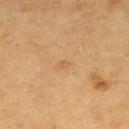Impression:
This lesion was catalogued during total-body skin photography and was not selected for biopsy.
Acquisition and patient details:
This is a cross-polarized tile. The patient is a female roughly 60 years of age. On the back. This image is a 15 mm lesion crop taken from a total-body photograph.